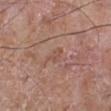Imaged during a routine full-body skin examination; the lesion was not biopsied and no histopathology is available.